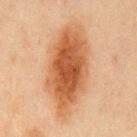Impression:
Recorded during total-body skin imaging; not selected for excision or biopsy.
Background:
The lesion is located on the chest. A lesion tile, about 15 mm wide, cut from a 3D total-body photograph. A male subject roughly 45 years of age.